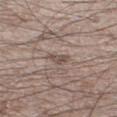follow-up = total-body-photography surveillance lesion; no biopsy
body site = the left thigh
automated lesion analysis = a symmetry-axis asymmetry near 0.4; a border-irregularity rating of about 4/10, a color-variation rating of about 2/10, and a peripheral color-asymmetry measure near 0.5
patient = male, aged 48 to 52
size = ~2.5 mm (longest diameter)
illumination = white-light illumination
image source = total-body-photography crop, ~15 mm field of view